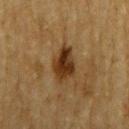Q: Is there a histopathology result?
A: catalogued during a skin exam; not biopsied
Q: How was this image acquired?
A: ~15 mm crop, total-body skin-cancer survey
Q: How was the tile lit?
A: cross-polarized
Q: Where on the body is the lesion?
A: the right upper arm
Q: Lesion size?
A: ≈4 mm
Q: Automated lesion metrics?
A: a lesion area of about 9 mm², an eccentricity of roughly 0.7, and two-axis asymmetry of about 0.3; a nevus-likeness score of about 90/100
Q: Patient demographics?
A: male, in their mid- to late 80s or older